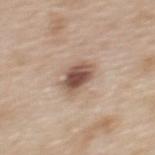patient: female, aged 48 to 52
image-analysis metrics: two-axis asymmetry of about 0.2; an average lesion color of about L≈53 a*≈18 b*≈26 (CIELAB), roughly 15 lightness units darker than nearby skin, and a normalized lesion–skin contrast near 10; a peripheral color-asymmetry measure near 1.5; a classifier nevus-likeness of about 90/100 and lesion-presence confidence of about 100/100
anatomic site: the upper back
image source: 15 mm crop, total-body photography
diameter: ≈3.5 mm